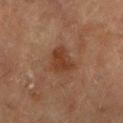Assessment:
Recorded during total-body skin imaging; not selected for excision or biopsy.
Background:
The lesion is located on the left lower leg. This image is a 15 mm lesion crop taken from a total-body photograph. A male subject, aged around 85 or older. The tile uses cross-polarized illumination.